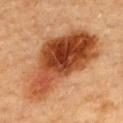| key | value |
|---|---|
| follow-up | total-body-photography surveillance lesion; no biopsy |
| tile lighting | cross-polarized |
| patient | female, approximately 60 years of age |
| size | ~11.5 mm (longest diameter) |
| anatomic site | the upper back |
| acquisition | total-body-photography crop, ~15 mm field of view |